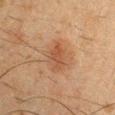Assessment: Imaged during a routine full-body skin examination; the lesion was not biopsied and no histopathology is available. Background: The lesion is located on the right upper arm. A male patient aged around 35. An algorithmic analysis of the crop reported a border-irregularity index near 2.5/10, a color-variation rating of about 3/10, and a peripheral color-asymmetry measure near 1. The recorded lesion diameter is about 4 mm. A close-up tile cropped from a whole-body skin photograph, about 15 mm across.